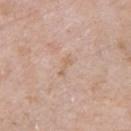Recorded during total-body skin imaging; not selected for excision or biopsy. Imaged with white-light lighting. A roughly 15 mm field-of-view crop from a total-body skin photograph. The total-body-photography lesion software estimated a lesion–skin lightness drop of about 6. It also reported border irregularity of about 5 on a 0–10 scale, a within-lesion color-variation index near 0/10, and peripheral color asymmetry of about 0. It also reported an automated nevus-likeness rating near 0 out of 100 and lesion-presence confidence of about 100/100. A female patient, aged around 85 or older. On the chest.